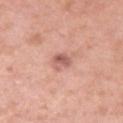The lesion was photographed on a routine skin check and not biopsied; there is no pathology result.
From the left upper arm.
A female subject, about 40 years old.
A region of skin cropped from a whole-body photographic capture, roughly 15 mm wide.
The tile uses white-light illumination.
Automated tile analysis of the lesion measured a shape eccentricity near 0.7. The software also gave an average lesion color of about L≈59 a*≈25 b*≈26 (CIELAB), about 12 CIELAB-L* units darker than the surrounding skin, and a normalized border contrast of about 7.5. The software also gave border irregularity of about 3.5 on a 0–10 scale, internal color variation of about 3 on a 0–10 scale, and radial color variation of about 1. The analysis additionally found a classifier nevus-likeness of about 35/100 and a lesion-detection confidence of about 100/100.
Measured at roughly 3 mm in maximum diameter.